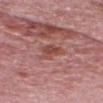follow-up: no biopsy performed (imaged during a skin exam); illumination: white-light illumination; patient: male, in their mid- to late 60s; body site: the head or neck; image source: total-body-photography crop, ~15 mm field of view.